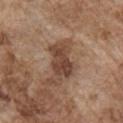follow-up — imaged on a skin check; not biopsied
image source — 15 mm crop, total-body photography
subject — male, aged around 75
size — about 4.5 mm
tile lighting — white-light
anatomic site — the right upper arm